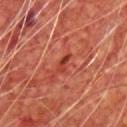The lesion was tiled from a total-body skin photograph and was not biopsied. A male subject aged approximately 65. The lesion is located on the front of the torso. A 15 mm close-up extracted from a 3D total-body photography capture.